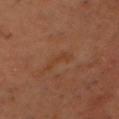notes = imaged on a skin check; not biopsied
automated metrics = a normalized border contrast of about 4.5; a border-irregularity rating of about 5/10, a color-variation rating of about 0.5/10, and a peripheral color-asymmetry measure near 0; a nevus-likeness score of about 0/100 and lesion-presence confidence of about 100/100
location = the head or neck
subject = male, aged 53 to 57
lighting = cross-polarized
image source = 15 mm crop, total-body photography